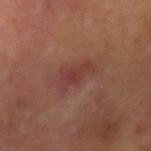Imaged during a routine full-body skin examination; the lesion was not biopsied and no histopathology is available.
About 4 mm across.
An algorithmic analysis of the crop reported a lesion area of about 6.5 mm², an outline eccentricity of about 0.85 (0 = round, 1 = elongated), and a shape-asymmetry score of about 0.4 (0 = symmetric). The analysis additionally found a border-irregularity index near 4.5/10, internal color variation of about 2.5 on a 0–10 scale, and a peripheral color-asymmetry measure near 1. The software also gave a nevus-likeness score of about 25/100 and a detector confidence of about 100 out of 100 that the crop contains a lesion.
Located on the right upper arm.
This is a cross-polarized tile.
A male subject aged around 70.
Cropped from a whole-body photographic skin survey; the tile spans about 15 mm.